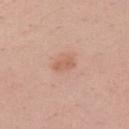biopsy status: total-body-photography surveillance lesion; no biopsy
illumination: white-light
image source: total-body-photography crop, ~15 mm field of view
diameter: ~2.5 mm (longest diameter)
body site: the left upper arm
image-analysis metrics: an average lesion color of about L≈60 a*≈23 b*≈31 (CIELAB), a lesion–skin lightness drop of about 8, and a normalized border contrast of about 6; a border-irregularity rating of about 3/10, internal color variation of about 1 on a 0–10 scale, and radial color variation of about 0.5; lesion-presence confidence of about 100/100
patient: female, in their mid- to late 20s